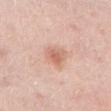biopsy status: total-body-photography surveillance lesion; no biopsy
subject: female, aged around 45
acquisition: 15 mm crop, total-body photography
anatomic site: the left lower leg